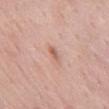– follow-up — total-body-photography surveillance lesion; no biopsy
– site — the front of the torso
– subject — male, in their mid-50s
– image — ~15 mm crop, total-body skin-cancer survey
– illumination — white-light illumination